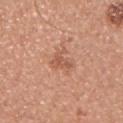A lesion tile, about 15 mm wide, cut from a 3D total-body photograph. Located on the chest. A male subject approximately 30 years of age.